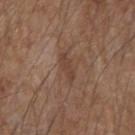Clinical impression:
Part of a total-body skin-imaging series; this lesion was reviewed on a skin check and was not flagged for biopsy.
Clinical summary:
A 15 mm close-up tile from a total-body photography series done for melanoma screening. Automated image analysis of the tile measured an area of roughly 4 mm², an outline eccentricity of about 0.9 (0 = round, 1 = elongated), and a symmetry-axis asymmetry near 0.25. It also reported a border-irregularity rating of about 3.5/10, a color-variation rating of about 1/10, and a peripheral color-asymmetry measure near 0. And it measured a nevus-likeness score of about 0/100 and a lesion-detection confidence of about 100/100. Approximately 3 mm at its widest. A male patient, aged around 55. Captured under white-light illumination. Located on the left forearm.